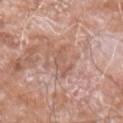Q: Is there a histopathology result?
A: total-body-photography surveillance lesion; no biopsy
Q: What are the patient's age and sex?
A: male, aged around 60
Q: What is the anatomic site?
A: the left forearm
Q: How was this image acquired?
A: ~15 mm tile from a whole-body skin photo
Q: Automated lesion metrics?
A: a lesion–skin lightness drop of about 7 and a lesion-to-skin contrast of about 5 (normalized; higher = more distinct)